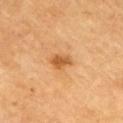Impression: Captured during whole-body skin photography for melanoma surveillance; the lesion was not biopsied. Context: A roughly 15 mm field-of-view crop from a total-body skin photograph. The subject is a male aged 68–72. Automated image analysis of the tile measured a lesion area of about 4.5 mm², an eccentricity of roughly 0.7, and a shape-asymmetry score of about 0.3 (0 = symmetric). Located on the right upper arm.